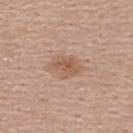Notes:
- notes: catalogued during a skin exam; not biopsied
- automated metrics: a footprint of about 7.5 mm², an eccentricity of roughly 0.75, and two-axis asymmetry of about 0.2; a lesion–skin lightness drop of about 9 and a normalized lesion–skin contrast near 6.5; lesion-presence confidence of about 100/100
- illumination: white-light illumination
- body site: the upper back
- size: ~4 mm (longest diameter)
- imaging modality: ~15 mm tile from a whole-body skin photo
- patient: female, aged approximately 60